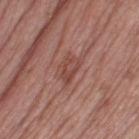Recorded during total-body skin imaging; not selected for excision or biopsy.
The lesion-visualizer software estimated an eccentricity of roughly 0.85 and two-axis asymmetry of about 0.35. The software also gave a border-irregularity rating of about 3.5/10, a color-variation rating of about 3.5/10, and radial color variation of about 1. The analysis additionally found an automated nevus-likeness rating near 0 out of 100 and a lesion-detection confidence of about 100/100.
Captured under white-light illumination.
On the leg.
A female patient approximately 55 years of age.
The lesion's longest dimension is about 3.5 mm.
This image is a 15 mm lesion crop taken from a total-body photograph.